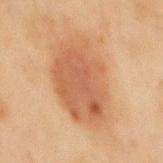Impression:
Recorded during total-body skin imaging; not selected for excision or biopsy.
Clinical summary:
The subject is a male in their mid-40s. A 15 mm crop from a total-body photograph taken for skin-cancer surveillance. Automated tile analysis of the lesion measured a symmetry-axis asymmetry near 0.15. It also reported roughly 9 lightness units darker than nearby skin and a lesion-to-skin contrast of about 7 (normalized; higher = more distinct). The software also gave a classifier nevus-likeness of about 95/100 and a lesion-detection confidence of about 100/100. Captured under cross-polarized illumination. The recorded lesion diameter is about 8.5 mm. The lesion is located on the mid back.